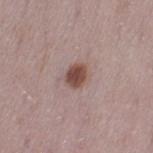Imaged during a routine full-body skin examination; the lesion was not biopsied and no histopathology is available. The lesion is on the left thigh. This image is a 15 mm lesion crop taken from a total-body photograph. This is a white-light tile. Approximately 2.5 mm at its widest. The patient is a female aged 28 to 32.